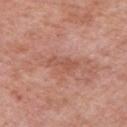<case>
  <biopsy_status>not biopsied; imaged during a skin examination</biopsy_status>
  <image>
    <source>total-body photography crop</source>
    <field_of_view_mm>15</field_of_view_mm>
  </image>
  <lesion_size>
    <long_diameter_mm_approx>3.5</long_diameter_mm_approx>
  </lesion_size>
  <site>left upper arm</site>
  <lighting>white-light</lighting>
  <patient>
    <sex>male</sex>
    <age_approx>55</age_approx>
  </patient>
</case>